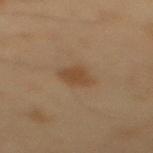<lesion>
<biopsy_status>not biopsied; imaged during a skin examination</biopsy_status>
</lesion>